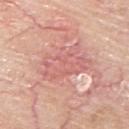Clinical impression: Captured during whole-body skin photography for melanoma surveillance; the lesion was not biopsied. Image and clinical context: Cropped from a whole-body photographic skin survey; the tile spans about 15 mm. The lesion is located on the back. A male subject, in their mid-60s.